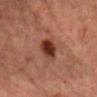The lesion was tiled from a total-body skin photograph and was not biopsied.
A female subject, approximately 45 years of age.
Automated tile analysis of the lesion measured a border-irregularity index near 2/10 and a color-variation rating of about 5.5/10. The software also gave a nevus-likeness score of about 100/100 and a lesion-detection confidence of about 100/100.
From the head or neck.
Cropped from a total-body skin-imaging series; the visible field is about 15 mm.